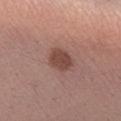Impression: This lesion was catalogued during total-body skin photography and was not selected for biopsy. Acquisition and patient details: Cropped from a whole-body photographic skin survey; the tile spans about 15 mm. The patient is a female about 25 years old. On the arm. The tile uses white-light illumination. The lesion's longest dimension is about 3.5 mm. Automated image analysis of the tile measured a border-irregularity index near 1.5/10, a color-variation rating of about 1.5/10, and peripheral color asymmetry of about 0.5. And it measured a classifier nevus-likeness of about 70/100.